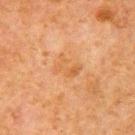| feature | finding |
|---|---|
| follow-up | total-body-photography surveillance lesion; no biopsy |
| illumination | cross-polarized illumination |
| lesion diameter | about 3 mm |
| imaging modality | ~15 mm crop, total-body skin-cancer survey |
| automated lesion analysis | an area of roughly 5.5 mm² and an eccentricity of roughly 0.75; a color-variation rating of about 3.5/10 and a peripheral color-asymmetry measure near 1 |
| body site | the right upper arm |
| patient | male, in their 80s |